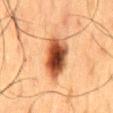<record>
  <patient>
    <sex>male</sex>
    <age_approx>60</age_approx>
  </patient>
  <site>mid back</site>
  <automated_metrics>
    <cielab_L>41</cielab_L>
    <cielab_a>23</cielab_a>
    <cielab_b>32</cielab_b>
    <vs_skin_darker_L>18.0</vs_skin_darker_L>
    <vs_skin_contrast_norm>13.0</vs_skin_contrast_norm>
    <border_irregularity_0_10>2.0</border_irregularity_0_10>
    <color_variation_0_10>9.0</color_variation_0_10>
    <peripheral_color_asymmetry>2.5</peripheral_color_asymmetry>
  </automated_metrics>
  <lighting>cross-polarized</lighting>
  <lesion_size>
    <long_diameter_mm_approx>6.0</long_diameter_mm_approx>
  </lesion_size>
  <image>
    <source>total-body photography crop</source>
    <field_of_view_mm>15</field_of_view_mm>
  </image>
</record>